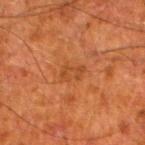Captured during whole-body skin photography for melanoma surveillance; the lesion was not biopsied. The lesion is on the leg. Cropped from a total-body skin-imaging series; the visible field is about 15 mm. A male subject roughly 80 years of age.